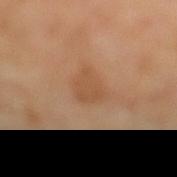Recorded during total-body skin imaging; not selected for excision or biopsy. A female patient aged approximately 50. The lesion is located on the left lower leg. A region of skin cropped from a whole-body photographic capture, roughly 15 mm wide.